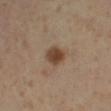The lesion was photographed on a routine skin check and not biopsied; there is no pathology result. The lesion's longest dimension is about 2.5 mm. Cropped from a total-body skin-imaging series; the visible field is about 15 mm. Captured under cross-polarized illumination. On the left lower leg. A female patient, aged approximately 40.